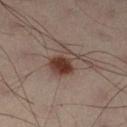| feature | finding |
|---|---|
| follow-up | total-body-photography surveillance lesion; no biopsy |
| acquisition | ~15 mm crop, total-body skin-cancer survey |
| anatomic site | the leg |
| patient | male, aged 38–42 |